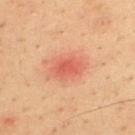Case summary:
* biopsy status: total-body-photography surveillance lesion; no biopsy
* lesion diameter: ≈4 mm
* subject: male, roughly 35 years of age
* body site: the back
* image source: ~15 mm tile from a whole-body skin photo
* image-analysis metrics: an area of roughly 8 mm², an outline eccentricity of about 0.75 (0 = round, 1 = elongated), and a symmetry-axis asymmetry near 0.25; a within-lesion color-variation index near 2.5/10 and radial color variation of about 1
* tile lighting: cross-polarized